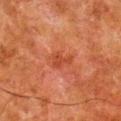Image and clinical context:
A 15 mm close-up extracted from a 3D total-body photography capture. Captured under cross-polarized illumination. On the right lower leg. The total-body-photography lesion software estimated a lesion-detection confidence of about 100/100. The patient is a male about 80 years old. Measured at roughly 3 mm in maximum diameter.